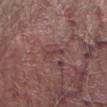Assessment: Part of a total-body skin-imaging series; this lesion was reviewed on a skin check and was not flagged for biopsy. Clinical summary: Automated tile analysis of the lesion measured an area of roughly 2 mm², an outline eccentricity of about 0.95 (0 = round, 1 = elongated), and two-axis asymmetry of about 0.5. The analysis additionally found a lesion color around L≈40 a*≈24 b*≈19 in CIELAB and a lesion–skin lightness drop of about 6. This is a white-light tile. A male subject, in their mid- to late 60s. The lesion's longest dimension is about 3 mm. A 15 mm close-up extracted from a 3D total-body photography capture. The lesion is located on the leg.